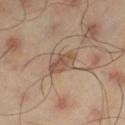Assessment: Imaged during a routine full-body skin examination; the lesion was not biopsied and no histopathology is available. Image and clinical context: Imaged with cross-polarized lighting. Located on the left thigh. A 15 mm close-up extracted from a 3D total-body photography capture. A male patient, in their mid-40s. The total-body-photography lesion software estimated a lesion area of about 8.5 mm², a shape eccentricity near 0.8, and two-axis asymmetry of about 0.2. And it measured an average lesion color of about L≈52 a*≈16 b*≈27 (CIELAB), a lesion–skin lightness drop of about 9, and a normalized border contrast of about 6.5. The lesion's longest dimension is about 4.5 mm.